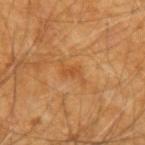Case summary:
* follow-up · catalogued during a skin exam; not biopsied
* image source · 15 mm crop, total-body photography
* automated lesion analysis · border irregularity of about 6 on a 0–10 scale and a peripheral color-asymmetry measure near 0; an automated nevus-likeness rating near 0 out of 100 and a lesion-detection confidence of about 100/100
* anatomic site · the left forearm
* subject · male, roughly 60 years of age
* tile lighting · cross-polarized
* lesion diameter · ≈3 mm